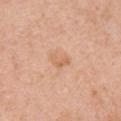<lesion>
  <image>
    <source>total-body photography crop</source>
    <field_of_view_mm>15</field_of_view_mm>
  </image>
  <patient>
    <sex>female</sex>
    <age_approx>40</age_approx>
  </patient>
  <site>right upper arm</site>
  <lighting>white-light</lighting>
  <lesion_size>
    <long_diameter_mm_approx>2.5</long_diameter_mm_approx>
  </lesion_size>
</lesion>